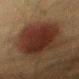Findings:
– biopsy status · total-body-photography surveillance lesion; no biopsy
– acquisition · ~15 mm tile from a whole-body skin photo
– automated lesion analysis · an outline eccentricity of about 0.65 (0 = round, 1 = elongated) and two-axis asymmetry of about 0.15; a border-irregularity index near 2/10 and internal color variation of about 4 on a 0–10 scale
– body site · the arm
– subject · male, in their mid-30s
– diameter · ≈7 mm